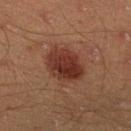workup=no biopsy performed (imaged during a skin exam) | subject=male, aged 43–47 | illumination=cross-polarized illumination | automated metrics=a lesion area of about 14 mm², a shape eccentricity near 0.65, and two-axis asymmetry of about 0.15; an automated nevus-likeness rating near 95 out of 100 | size=about 5 mm | location=the leg | image=total-body-photography crop, ~15 mm field of view.